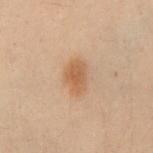Q: Is there a histopathology result?
A: total-body-photography surveillance lesion; no biopsy